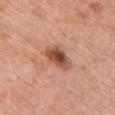Q: Is there a histopathology result?
A: total-body-photography surveillance lesion; no biopsy
Q: What is the anatomic site?
A: the chest
Q: What are the patient's age and sex?
A: female, aged 58 to 62
Q: What kind of image is this?
A: ~15 mm tile from a whole-body skin photo